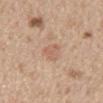Clinical impression:
Captured during whole-body skin photography for melanoma surveillance; the lesion was not biopsied.
Image and clinical context:
On the mid back. Automated image analysis of the tile measured a lesion color around L≈60 a*≈20 b*≈29 in CIELAB, about 7 CIELAB-L* units darker than the surrounding skin, and a lesion-to-skin contrast of about 4.5 (normalized; higher = more distinct). A male patient, aged 68–72. Captured under white-light illumination. A 15 mm close-up extracted from a 3D total-body photography capture. The recorded lesion diameter is about 3 mm.